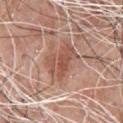The lesion was photographed on a routine skin check and not biopsied; there is no pathology result.
A male subject, aged 58 to 62.
From the chest.
Cropped from a total-body skin-imaging series; the visible field is about 15 mm.
The lesion-visualizer software estimated a footprint of about 10 mm², an eccentricity of roughly 0.75, and a shape-asymmetry score of about 0.45 (0 = symmetric). It also reported an average lesion color of about L≈53 a*≈23 b*≈28 (CIELAB) and about 10 CIELAB-L* units darker than the surrounding skin. And it measured a classifier nevus-likeness of about 0/100.